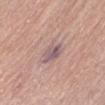workup: no biopsy performed (imaged during a skin exam)
tile lighting: white-light
patient: female, in their mid- to late 70s
TBP lesion metrics: a mean CIELAB color near L≈56 a*≈18 b*≈17, about 9 CIELAB-L* units darker than the surrounding skin, and a normalized border contrast of about 7.5; border irregularity of about 2 on a 0–10 scale and radial color variation of about 2; an automated nevus-likeness rating near 0 out of 100 and lesion-presence confidence of about 75/100
diameter: ~3 mm (longest diameter)
body site: the abdomen
image: ~15 mm tile from a whole-body skin photo